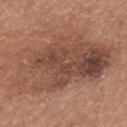Q: Was this lesion biopsied?
A: catalogued during a skin exam; not biopsied
Q: Lesion location?
A: the mid back
Q: What did automated image analysis measure?
A: a footprint of about 55 mm², a shape eccentricity near 0.85, and two-axis asymmetry of about 0.3; border irregularity of about 6.5 on a 0–10 scale and internal color variation of about 8.5 on a 0–10 scale; a classifier nevus-likeness of about 15/100 and a detector confidence of about 100 out of 100 that the crop contains a lesion
Q: Who is the patient?
A: male, in their mid-60s
Q: What is the imaging modality?
A: 15 mm crop, total-body photography
Q: Illumination type?
A: white-light illumination
Q: What is the lesion's diameter?
A: ~12.5 mm (longest diameter)